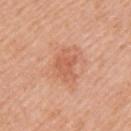notes=total-body-photography surveillance lesion; no biopsy
site=the arm
diameter=~2.5 mm (longest diameter)
image source=~15 mm tile from a whole-body skin photo
patient=female, approximately 40 years of age